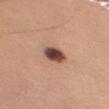Q: Where on the body is the lesion?
A: the arm
Q: What are the patient's age and sex?
A: female, aged approximately 45
Q: How was this image acquired?
A: 15 mm crop, total-body photography